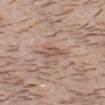No biopsy was performed on this lesion — it was imaged during a full skin examination and was not determined to be concerning.
The lesion is located on the head or neck.
Longest diameter approximately 3 mm.
Captured under white-light illumination.
A male patient, aged around 30.
A region of skin cropped from a whole-body photographic capture, roughly 15 mm wide.
Automated tile analysis of the lesion measured two-axis asymmetry of about 0.4. The analysis additionally found internal color variation of about 2 on a 0–10 scale and radial color variation of about 0.5.